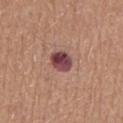This lesion was catalogued during total-body skin photography and was not selected for biopsy.
A 15 mm close-up tile from a total-body photography series done for melanoma screening.
Automated tile analysis of the lesion measured an area of roughly 6.5 mm² and two-axis asymmetry of about 0.1. It also reported a border-irregularity index near 1/10, internal color variation of about 7 on a 0–10 scale, and radial color variation of about 2.5. And it measured a classifier nevus-likeness of about 30/100.
The patient is a male approximately 65 years of age.
The tile uses white-light illumination.
Approximately 3 mm at its widest.
The lesion is on the mid back.